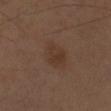Case summary:
* biopsy status · total-body-photography surveillance lesion; no biopsy
* tile lighting · cross-polarized
* location · the left forearm
* patient · male, aged around 70
* lesion diameter · ~3.5 mm (longest diameter)
* image-analysis metrics · an outline eccentricity of about 0.7 (0 = round, 1 = elongated) and two-axis asymmetry of about 0.25; a lesion color around L≈33 a*≈16 b*≈24 in CIELAB and a lesion-to-skin contrast of about 6 (normalized; higher = more distinct); a within-lesion color-variation index near 2/10 and peripheral color asymmetry of about 1; a classifier nevus-likeness of about 30/100 and lesion-presence confidence of about 100/100
* acquisition · 15 mm crop, total-body photography